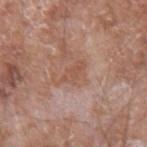Part of a total-body skin-imaging series; this lesion was reviewed on a skin check and was not flagged for biopsy. Imaged with white-light lighting. The subject is a male aged around 60. The lesion-visualizer software estimated an area of roughly 5 mm², an eccentricity of roughly 0.85, and a symmetry-axis asymmetry near 0.4. The software also gave an average lesion color of about L≈53 a*≈21 b*≈29 (CIELAB) and a normalized lesion–skin contrast near 5.5. And it measured border irregularity of about 4.5 on a 0–10 scale, a color-variation rating of about 1/10, and a peripheral color-asymmetry measure near 0.5. A region of skin cropped from a whole-body photographic capture, roughly 15 mm wide. The lesion is on the arm. Longest diameter approximately 4 mm.